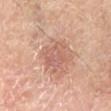Impression:
This lesion was catalogued during total-body skin photography and was not selected for biopsy.
Clinical summary:
A 15 mm close-up tile from a total-body photography series done for melanoma screening. A male subject in their mid-60s. From the leg.